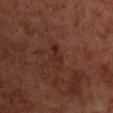image = ~15 mm tile from a whole-body skin photo; anatomic site = the front of the torso; subject = male, approximately 70 years of age.